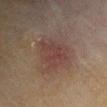| field | value |
|---|---|
| workup | total-body-photography surveillance lesion; no biopsy |
| acquisition | total-body-photography crop, ~15 mm field of view |
| tile lighting | cross-polarized illumination |
| patient | male, approximately 70 years of age |
| lesion size | ~6.5 mm (longest diameter) |
| site | the left upper arm |
| automated lesion analysis | a lesion area of about 23 mm² and a shape eccentricity near 0.7; about 5 CIELAB-L* units darker than the surrounding skin and a normalized border contrast of about 5.5; border irregularity of about 3 on a 0–10 scale and radial color variation of about 1; a lesion-detection confidence of about 100/100 |